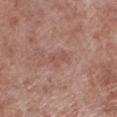| field | value |
|---|---|
| workup | total-body-photography surveillance lesion; no biopsy |
| image source | 15 mm crop, total-body photography |
| site | the left lower leg |
| illumination | white-light illumination |
| subject | male, approximately 55 years of age |
| size | ≈2.5 mm |